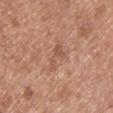The lesion was photographed on a routine skin check and not biopsied; there is no pathology result. The patient is a female in their 40s. A 15 mm close-up extracted from a 3D total-body photography capture. The lesion is on the front of the torso.